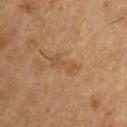No biopsy was performed on this lesion — it was imaged during a full skin examination and was not determined to be concerning. Automated tile analysis of the lesion measured a mean CIELAB color near L≈49 a*≈19 b*≈34, a lesion–skin lightness drop of about 6, and a normalized border contrast of about 5. The analysis additionally found a border-irregularity index near 4.5/10, a color-variation rating of about 5.5/10, and radial color variation of about 2. The analysis additionally found an automated nevus-likeness rating near 0 out of 100. From the right upper arm. Longest diameter approximately 4 mm. A lesion tile, about 15 mm wide, cut from a 3D total-body photograph. Captured under cross-polarized illumination. The subject is a male aged 53–57.